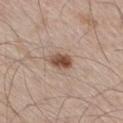{
  "site": "right thigh",
  "lighting": "white-light",
  "image": {
    "source": "total-body photography crop",
    "field_of_view_mm": 15
  },
  "lesion_size": {
    "long_diameter_mm_approx": 3.0
  },
  "automated_metrics": {
    "cielab_L": 50,
    "cielab_a": 19,
    "cielab_b": 27,
    "vs_skin_darker_L": 15.0,
    "vs_skin_contrast_norm": 10.5
  },
  "patient": {
    "sex": "male",
    "age_approx": 60
  }
}